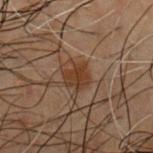Clinical impression:
Recorded during total-body skin imaging; not selected for excision or biopsy.
Acquisition and patient details:
The lesion is on the chest. An algorithmic analysis of the crop reported a lesion area of about 5 mm² and a shape-asymmetry score of about 0.35 (0 = symmetric). The tile uses cross-polarized illumination. A 15 mm close-up tile from a total-body photography series done for melanoma screening. A male subject in their 50s.